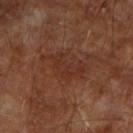Acquisition and patient details:
A roughly 15 mm field-of-view crop from a total-body skin photograph. Measured at roughly 5.5 mm in maximum diameter. A male patient, aged around 65. This is a cross-polarized tile. The lesion-visualizer software estimated a lesion area of about 8.5 mm² and an eccentricity of roughly 0.85. It also reported a lesion color around L≈30 a*≈22 b*≈26 in CIELAB and a normalized lesion–skin contrast near 5.5. The analysis additionally found a border-irregularity rating of about 7/10, a within-lesion color-variation index near 2/10, and radial color variation of about 0.5. The software also gave an automated nevus-likeness rating near 0 out of 100 and a lesion-detection confidence of about 100/100. On the right upper arm.